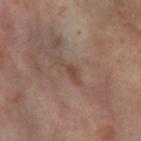Clinical impression: Recorded during total-body skin imaging; not selected for excision or biopsy. Background: On the right thigh. A 15 mm close-up extracted from a 3D total-body photography capture. The total-body-photography lesion software estimated an area of roughly 3 mm², an outline eccentricity of about 0.95 (0 = round, 1 = elongated), and a symmetry-axis asymmetry near 0.5. The software also gave an average lesion color of about L≈46 a*≈18 b*≈25 (CIELAB), about 7 CIELAB-L* units darker than the surrounding skin, and a normalized lesion–skin contrast near 6. The analysis additionally found border irregularity of about 6 on a 0–10 scale, a color-variation rating of about 0.5/10, and a peripheral color-asymmetry measure near 0. The analysis additionally found an automated nevus-likeness rating near 0 out of 100 and lesion-presence confidence of about 100/100. Longest diameter approximately 3.5 mm. The patient is a female about 55 years old.